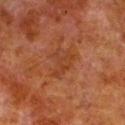The lesion was tiled from a total-body skin photograph and was not biopsied. The total-body-photography lesion software estimated a normalized border contrast of about 5. The analysis additionally found a lesion-detection confidence of about 100/100. Longest diameter approximately 4 mm. A male patient approximately 80 years of age. On the left lower leg. A 15 mm close-up tile from a total-body photography series done for melanoma screening. This is a cross-polarized tile.